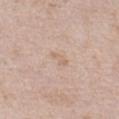Captured during whole-body skin photography for melanoma surveillance; the lesion was not biopsied.
This is a white-light tile.
A male subject, aged 48 to 52.
This image is a 15 mm lesion crop taken from a total-body photograph.
On the chest.